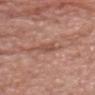Q: What is the lesion's diameter?
A: ≈2.5 mm
Q: What kind of image is this?
A: 15 mm crop, total-body photography
Q: Who is the patient?
A: male, aged 53–57